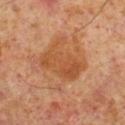No biopsy was performed on this lesion — it was imaged during a full skin examination and was not determined to be concerning.
The lesion is on the right lower leg.
The subject is a male approximately 60 years of age.
A 15 mm close-up extracted from a 3D total-body photography capture.